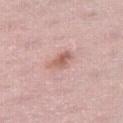Clinical impression: Imaged during a routine full-body skin examination; the lesion was not biopsied and no histopathology is available. Image and clinical context: Captured under white-light illumination. Automated tile analysis of the lesion measured a lesion–skin lightness drop of about 10 and a normalized lesion–skin contrast near 7. It also reported internal color variation of about 5 on a 0–10 scale. The analysis additionally found a nevus-likeness score of about 25/100 and lesion-presence confidence of about 100/100. A region of skin cropped from a whole-body photographic capture, roughly 15 mm wide. The patient is a female aged approximately 40. Located on the right thigh. Approximately 3.5 mm at its widest.